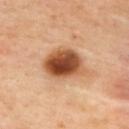The lesion was tiled from a total-body skin photograph and was not biopsied. On the upper back. Captured under cross-polarized illumination. The patient is a female aged approximately 40. Cropped from a whole-body photographic skin survey; the tile spans about 15 mm.